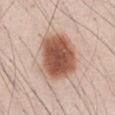No biopsy was performed on this lesion — it was imaged during a full skin examination and was not determined to be concerning. The lesion's longest dimension is about 6.5 mm. Cropped from a total-body skin-imaging series; the visible field is about 15 mm. Captured under white-light illumination. An algorithmic analysis of the crop reported a lesion area of about 26 mm² and an eccentricity of roughly 0.6. The software also gave a border-irregularity rating of about 2/10, a color-variation rating of about 5.5/10, and peripheral color asymmetry of about 1.5. It also reported an automated nevus-likeness rating near 100 out of 100 and a detector confidence of about 100 out of 100 that the crop contains a lesion. On the abdomen. A male subject, in their mid- to late 30s.